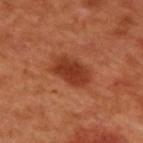{"lighting": "cross-polarized", "site": "upper back", "patient": {"sex": "male", "age_approx": 50}, "automated_metrics": {"area_mm2_approx": 10.0, "eccentricity": 0.8, "shape_asymmetry": 0.2, "cielab_L": 38, "cielab_a": 30, "cielab_b": 35, "vs_skin_contrast_norm": 9.0, "border_irregularity_0_10": 2.5}, "image": {"source": "total-body photography crop", "field_of_view_mm": 15}}